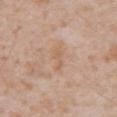<case>
<biopsy_status>not biopsied; imaged during a skin examination</biopsy_status>
<image>
  <source>total-body photography crop</source>
  <field_of_view_mm>15</field_of_view_mm>
</image>
<automated_metrics>
  <eccentricity>0.95</eccentricity>
  <shape_asymmetry>0.45</shape_asymmetry>
  <color_variation_0_10>0.0</color_variation_0_10>
  <peripheral_color_asymmetry>0.0</peripheral_color_asymmetry>
  <nevus_likeness_0_100>0</nevus_likeness_0_100>
</automated_metrics>
<site>front of the torso</site>
<lesion_size>
  <long_diameter_mm_approx>3.0</long_diameter_mm_approx>
</lesion_size>
<lighting>white-light</lighting>
<patient>
  <sex>male</sex>
  <age_approx>65</age_approx>
</patient>
</case>